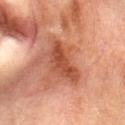follow-up: catalogued during a skin exam; not biopsied | acquisition: ~15 mm tile from a whole-body skin photo | body site: the abdomen | illumination: cross-polarized | image-analysis metrics: an eccentricity of roughly 0.9 and a symmetry-axis asymmetry near 0.4; a border-irregularity rating of about 4/10, a within-lesion color-variation index near 4/10, and a peripheral color-asymmetry measure near 1.5; a classifier nevus-likeness of about 0/100 and lesion-presence confidence of about 100/100 | subject: female, approximately 30 years of age.